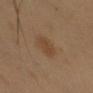This lesion was catalogued during total-body skin photography and was not selected for biopsy.
The subject is a male aged around 50.
Approximately 3.5 mm at its widest.
The lesion-visualizer software estimated a footprint of about 5.5 mm², an outline eccentricity of about 0.85 (0 = round, 1 = elongated), and a symmetry-axis asymmetry near 0.15. The analysis additionally found a border-irregularity rating of about 2/10, a color-variation rating of about 1.5/10, and radial color variation of about 0.5. The analysis additionally found a nevus-likeness score of about 65/100 and a detector confidence of about 100 out of 100 that the crop contains a lesion.
On the left upper arm.
This is a cross-polarized tile.
Cropped from a total-body skin-imaging series; the visible field is about 15 mm.